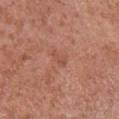notes=imaged on a skin check; not biopsied